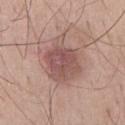Captured during whole-body skin photography for melanoma surveillance; the lesion was not biopsied. Imaged with white-light lighting. Located on the chest. A male patient aged 63–67. A region of skin cropped from a whole-body photographic capture, roughly 15 mm wide.